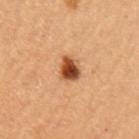notes = catalogued during a skin exam; not biopsied | size = about 3 mm | acquisition = total-body-photography crop, ~15 mm field of view | subject = female, roughly 50 years of age | body site = the left upper arm.